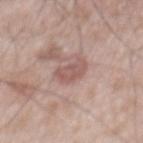Assessment:
The lesion was tiled from a total-body skin photograph and was not biopsied.
Image and clinical context:
A 15 mm close-up tile from a total-body photography series done for melanoma screening. The lesion is located on the mid back. Measured at roughly 3 mm in maximum diameter. The subject is a male aged 48–52.